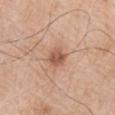biopsy status — catalogued during a skin exam; not biopsied
patient — male, aged 73 to 77
TBP lesion metrics — an eccentricity of roughly 0.45 and a symmetry-axis asymmetry near 0.2; a border-irregularity index near 2/10, a within-lesion color-variation index near 3.5/10, and radial color variation of about 1.5; a nevus-likeness score of about 75/100 and lesion-presence confidence of about 100/100
location — the right upper arm
acquisition — ~15 mm crop, total-body skin-cancer survey
lesion diameter — ≈2.5 mm
illumination — white-light